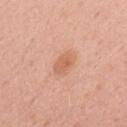– biopsy status — catalogued during a skin exam; not biopsied
– site — the upper back
– imaging modality — 15 mm crop, total-body photography
– lesion diameter — ≈3 mm
– subject — male, in their mid-50s
– TBP lesion metrics — border irregularity of about 2 on a 0–10 scale; a nevus-likeness score of about 50/100